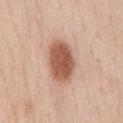Notes:
– lesion diameter — ≈5 mm
– patient — male, roughly 50 years of age
– image source — 15 mm crop, total-body photography
– lighting — white-light
– TBP lesion metrics — a lesion area of about 14 mm², an eccentricity of roughly 0.8, and two-axis asymmetry of about 0.15; a mean CIELAB color near L≈56 a*≈24 b*≈30, a lesion–skin lightness drop of about 16, and a normalized border contrast of about 10.5; a within-lesion color-variation index near 4/10 and peripheral color asymmetry of about 1
– site — the chest